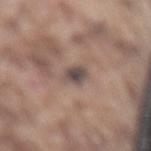follow-up: imaged on a skin check; not biopsied
lighting: white-light illumination
imaging modality: ~15 mm crop, total-body skin-cancer survey
TBP lesion metrics: a footprint of about 4.5 mm², an eccentricity of roughly 0.75, and a shape-asymmetry score of about 0.25 (0 = symmetric)
diameter: about 3 mm
body site: the back
patient: male, roughly 75 years of age